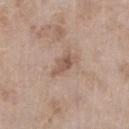{"biopsy_status": "not biopsied; imaged during a skin examination", "automated_metrics": {"cielab_L": 54, "cielab_a": 17, "cielab_b": 27, "vs_skin_darker_L": 10.0, "color_variation_0_10": 2.5}, "site": "right lower leg", "patient": {"sex": "female", "age_approx": 75}, "lesion_size": {"long_diameter_mm_approx": 3.5}, "image": {"source": "total-body photography crop", "field_of_view_mm": 15}}